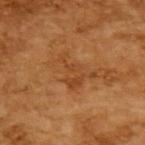notes — imaged on a skin check; not biopsied
TBP lesion metrics — a mean CIELAB color near L≈43 a*≈24 b*≈39, a lesion–skin lightness drop of about 7, and a lesion-to-skin contrast of about 5.5 (normalized; higher = more distinct); internal color variation of about 1.5 on a 0–10 scale and a peripheral color-asymmetry measure near 0.5; a classifier nevus-likeness of about 0/100
image source — 15 mm crop, total-body photography
lesion diameter — ≈4 mm
illumination — cross-polarized illumination
patient — male, in their mid- to late 60s